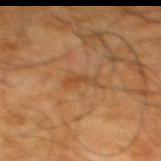{"biopsy_status": "not biopsied; imaged during a skin examination", "lighting": "cross-polarized", "lesion_size": {"long_diameter_mm_approx": 3.5}, "patient": {"sex": "male", "age_approx": 65}, "site": "upper back", "image": {"source": "total-body photography crop", "field_of_view_mm": 15}}